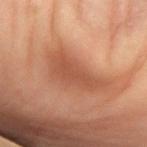Clinical impression:
Captured during whole-body skin photography for melanoma surveillance; the lesion was not biopsied.
Image and clinical context:
The recorded lesion diameter is about 7 mm. The lesion is on the left forearm. A female subject aged approximately 50. Automated tile analysis of the lesion measured a lesion area of about 18 mm² and a shape eccentricity near 0.9. The software also gave a lesion color around L≈53 a*≈25 b*≈33 in CIELAB and about 10 CIELAB-L* units darker than the surrounding skin. It also reported border irregularity of about 4.5 on a 0–10 scale, a color-variation rating of about 2.5/10, and a peripheral color-asymmetry measure near 0.5. Cropped from a total-body skin-imaging series; the visible field is about 15 mm.